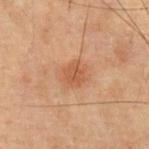  biopsy_status: not biopsied; imaged during a skin examination
  patient:
    sex: male
    age_approx: 70
  image:
    source: total-body photography crop
    field_of_view_mm: 15
  lesion_size:
    long_diameter_mm_approx: 2.5
  site: chest
  automated_metrics:
    area_mm2_approx: 5.0
    eccentricity: 0.3
    shape_asymmetry: 0.1
    cielab_L: 41
    cielab_a: 19
    cielab_b: 29
    vs_skin_darker_L: 7.0
    vs_skin_contrast_norm: 6.5
    border_irregularity_0_10: 1.5
    color_variation_0_10: 1.5
    peripheral_color_asymmetry: 0.5
  lighting: cross-polarized